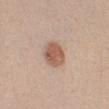Acquisition and patient details:
This is a white-light tile. From the chest. Longest diameter approximately 3 mm. A close-up tile cropped from a whole-body skin photograph, about 15 mm across. The subject is a female aged 23–27.